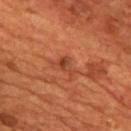Part of a total-body skin-imaging series; this lesion was reviewed on a skin check and was not flagged for biopsy. Automated tile analysis of the lesion measured a mean CIELAB color near L≈40 a*≈27 b*≈35 and a normalized lesion–skin contrast near 6. The analysis additionally found a border-irregularity rating of about 4.5/10, internal color variation of about 3.5 on a 0–10 scale, and peripheral color asymmetry of about 1. Approximately 2.5 mm at its widest. Captured under cross-polarized illumination. A male patient in their mid-50s. On the chest. Cropped from a total-body skin-imaging series; the visible field is about 15 mm.